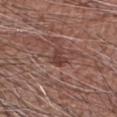Background: This is a white-light tile. Automated tile analysis of the lesion measured a footprint of about 3 mm², an eccentricity of roughly 0.8, and a shape-asymmetry score of about 0.3 (0 = symmetric). The analysis additionally found a lesion color around L≈40 a*≈21 b*≈24 in CIELAB and a normalized border contrast of about 7.5. It also reported a lesion-detection confidence of about 90/100. A close-up tile cropped from a whole-body skin photograph, about 15 mm across. Approximately 2.5 mm at its widest. Located on the right forearm. A male subject, aged 58 to 62.